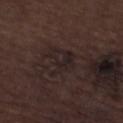Located on the left lower leg. A male subject aged 68 to 72. Automated tile analysis of the lesion measured a border-irregularity index near 6/10, a within-lesion color-variation index near 4.5/10, and a peripheral color-asymmetry measure near 1.5. A 15 mm crop from a total-body photograph taken for skin-cancer surveillance. About 4 mm across.